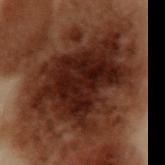follow-up = catalogued during a skin exam; not biopsied | body site = the left upper arm | subject = male, aged 53–57 | imaging modality = total-body-photography crop, ~15 mm field of view | lesion diameter = ~10.5 mm (longest diameter).